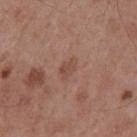follow-up = no biopsy performed (imaged during a skin exam) | tile lighting = white-light | acquisition = total-body-photography crop, ~15 mm field of view | body site = the mid back | subject = male, about 55 years old | automated metrics = an area of roughly 3 mm², an eccentricity of roughly 0.85, and a symmetry-axis asymmetry near 0.3; an average lesion color of about L≈48 a*≈21 b*≈28 (CIELAB), about 7 CIELAB-L* units darker than the surrounding skin, and a normalized border contrast of about 5.5.